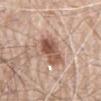Clinical impression: The lesion was photographed on a routine skin check and not biopsied; there is no pathology result. Clinical summary: Located on the back. Captured under white-light illumination. A 15 mm close-up extracted from a 3D total-body photography capture. The subject is a male about 80 years old. Approximately 5.5 mm at its widest.